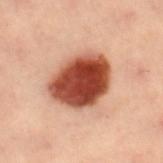Clinical impression: Imaged during a routine full-body skin examination; the lesion was not biopsied and no histopathology is available. Clinical summary: A female subject, aged around 60. A lesion tile, about 15 mm wide, cut from a 3D total-body photograph. On the left leg. The recorded lesion diameter is about 6 mm.